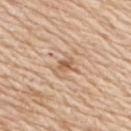The lesion was photographed on a routine skin check and not biopsied; there is no pathology result. The patient is a male aged approximately 60. Imaged with white-light lighting. A lesion tile, about 15 mm wide, cut from a 3D total-body photograph. Measured at roughly 3 mm in maximum diameter. Automated tile analysis of the lesion measured internal color variation of about 3 on a 0–10 scale and a peripheral color-asymmetry measure near 1. It also reported lesion-presence confidence of about 95/100. Located on the upper back.